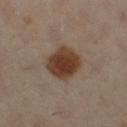Q: Was a biopsy performed?
A: total-body-photography surveillance lesion; no biopsy
Q: What are the patient's age and sex?
A: female, aged around 60
Q: Automated lesion metrics?
A: a lesion area of about 13 mm² and two-axis asymmetry of about 0.1; a lesion–skin lightness drop of about 11; a within-lesion color-variation index near 3.5/10 and peripheral color asymmetry of about 1
Q: What is the lesion's diameter?
A: ≈4.5 mm
Q: How was this image acquired?
A: ~15 mm crop, total-body skin-cancer survey
Q: Lesion location?
A: the right leg
Q: How was the tile lit?
A: cross-polarized